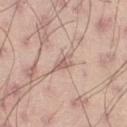Captured during whole-body skin photography for melanoma surveillance; the lesion was not biopsied. Cropped from a total-body skin-imaging series; the visible field is about 15 mm. A male patient approximately 45 years of age. The lesion is on the right thigh.